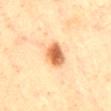Notes:
- biopsy status — total-body-photography surveillance lesion; no biopsy
- body site — the lower back
- imaging modality — total-body-photography crop, ~15 mm field of view
- subject — male, aged approximately 60
- lesion size — ~4 mm (longest diameter)